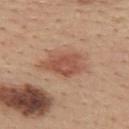<record>
<biopsy_status>not biopsied; imaged during a skin examination</biopsy_status>
</record>